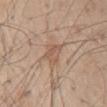Clinical impression: The lesion was photographed on a routine skin check and not biopsied; there is no pathology result. Acquisition and patient details: A male subject aged approximately 55. Located on the back. This image is a 15 mm lesion crop taken from a total-body photograph.